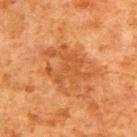notes: catalogued during a skin exam; not biopsied | size: about 6.5 mm | patient: male, aged around 80 | tile lighting: cross-polarized | image source: total-body-photography crop, ~15 mm field of view | site: the upper back | automated metrics: a footprint of about 22 mm² and a symmetry-axis asymmetry near 0.3.